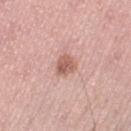Recorded during total-body skin imaging; not selected for excision or biopsy. Captured under white-light illumination. An algorithmic analysis of the crop reported a lesion area of about 4.5 mm², a shape eccentricity near 0.6, and two-axis asymmetry of about 0.25. And it measured a mean CIELAB color near L≈59 a*≈23 b*≈26, about 12 CIELAB-L* units darker than the surrounding skin, and a normalized lesion–skin contrast near 8. The analysis additionally found a border-irregularity index near 2/10 and internal color variation of about 3 on a 0–10 scale. The software also gave a classifier nevus-likeness of about 50/100 and a lesion-detection confidence of about 100/100. The lesion's longest dimension is about 2.5 mm. A lesion tile, about 15 mm wide, cut from a 3D total-body photograph. Located on the left thigh. The patient is a female aged 48 to 52.